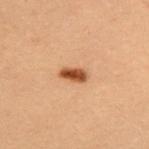<lesion>
  <biopsy_status>not biopsied; imaged during a skin examination</biopsy_status>
  <site>upper back</site>
  <lesion_size>
    <long_diameter_mm_approx>3.0</long_diameter_mm_approx>
  </lesion_size>
  <automated_metrics>
    <nevus_likeness_0_100>100</nevus_likeness_0_100>
    <lesion_detection_confidence_0_100>100</lesion_detection_confidence_0_100>
  </automated_metrics>
  <image>
    <source>total-body photography crop</source>
    <field_of_view_mm>15</field_of_view_mm>
  </image>
  <patient>
    <sex>female</sex>
    <age_approx>30</age_approx>
  </patient>
  <lighting>cross-polarized</lighting>
</lesion>